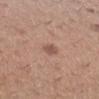Impression:
No biopsy was performed on this lesion — it was imaged during a full skin examination and was not determined to be concerning.
Clinical summary:
The lesion-visualizer software estimated a lesion area of about 6 mm² and a symmetry-axis asymmetry near 0.25. The analysis additionally found a mean CIELAB color near L≈56 a*≈19 b*≈26 and a lesion–skin lightness drop of about 7. Located on the leg. The lesion's longest dimension is about 3 mm. A roughly 15 mm field-of-view crop from a total-body skin photograph. The subject is a female aged approximately 30. This is a white-light tile.